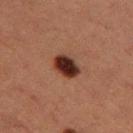Q: Was a biopsy performed?
A: catalogued during a skin exam; not biopsied
Q: How large is the lesion?
A: about 3.5 mm
Q: What did automated image analysis measure?
A: an area of roughly 6.5 mm², an outline eccentricity of about 0.7 (0 = round, 1 = elongated), and two-axis asymmetry of about 0.15; a lesion color around L≈25 a*≈20 b*≈22 in CIELAB and about 16 CIELAB-L* units darker than the surrounding skin
Q: What lighting was used for the tile?
A: cross-polarized
Q: What are the patient's age and sex?
A: female, in their 40s
Q: Lesion location?
A: the left thigh
Q: What kind of image is this?
A: total-body-photography crop, ~15 mm field of view